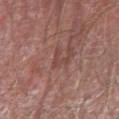{
  "biopsy_status": "not biopsied; imaged during a skin examination",
  "lesion_size": {
    "long_diameter_mm_approx": 3.0
  },
  "lighting": "white-light",
  "image": {
    "source": "total-body photography crop",
    "field_of_view_mm": 15
  },
  "site": "right forearm",
  "patient": {
    "sex": "male",
    "age_approx": 70
  }
}